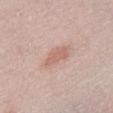Clinical impression:
No biopsy was performed on this lesion — it was imaged during a full skin examination and was not determined to be concerning.
Context:
From the chest. A female subject aged 63 to 67. Captured under white-light illumination. A roughly 15 mm field-of-view crop from a total-body skin photograph. Measured at roughly 3.5 mm in maximum diameter. The lesion-visualizer software estimated a lesion area of about 6 mm² and a symmetry-axis asymmetry near 0.3. The software also gave border irregularity of about 3.5 on a 0–10 scale, a within-lesion color-variation index near 1.5/10, and radial color variation of about 0.5.